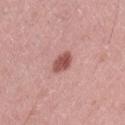<tbp_lesion>
<site>abdomen</site>
<lesion_size>
  <long_diameter_mm_approx>2.5</long_diameter_mm_approx>
</lesion_size>
<image>
  <source>total-body photography crop</source>
  <field_of_view_mm>15</field_of_view_mm>
</image>
<patient>
  <sex>male</sex>
  <age_approx>45</age_approx>
</patient>
<automated_metrics>
  <border_irregularity_0_10>2.0</border_irregularity_0_10>
  <color_variation_0_10>2.5</color_variation_0_10>
</automated_metrics>
</tbp_lesion>